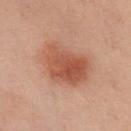workup = no biopsy performed (imaged during a skin exam); body site = the chest; image source = total-body-photography crop, ~15 mm field of view; subject = female, roughly 55 years of age.